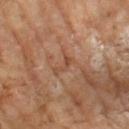Imaged during a routine full-body skin examination; the lesion was not biopsied and no histopathology is available. A male patient aged approximately 65. Cropped from a whole-body photographic skin survey; the tile spans about 15 mm.